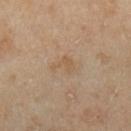{
  "biopsy_status": "not biopsied; imaged during a skin examination",
  "patient": {
    "sex": "female",
    "age_approx": 40
  },
  "site": "left forearm",
  "lighting": "cross-polarized",
  "image": {
    "source": "total-body photography crop",
    "field_of_view_mm": 15
  },
  "automated_metrics": {
    "cielab_L": 56,
    "cielab_a": 16,
    "cielab_b": 33,
    "vs_skin_darker_L": 5.0,
    "vs_skin_contrast_norm": 5.0,
    "nevus_likeness_0_100": 0,
    "lesion_detection_confidence_0_100": 100
  }
}